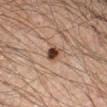<lesion>
<biopsy_status>not biopsied; imaged during a skin examination</biopsy_status>
</lesion>